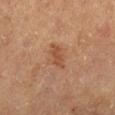{
  "biopsy_status": "not biopsied; imaged during a skin examination",
  "site": "right lower leg",
  "automated_metrics": {
    "area_mm2_approx": 3.5,
    "eccentricity": 0.85,
    "shape_asymmetry": 0.3
  },
  "lighting": "cross-polarized",
  "lesion_size": {
    "long_diameter_mm_approx": 3.0
  },
  "patient": {
    "sex": "male",
    "age_approx": "85+"
  },
  "image": {
    "source": "total-body photography crop",
    "field_of_view_mm": 15
  }
}